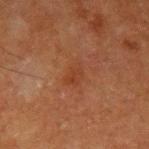Clinical impression: No biopsy was performed on this lesion — it was imaged during a full skin examination and was not determined to be concerning. Acquisition and patient details: A 15 mm close-up extracted from a 3D total-body photography capture. Imaged with cross-polarized lighting. A male patient aged around 75. Longest diameter approximately 3 mm. On the left upper arm. Automated tile analysis of the lesion measured a footprint of about 4 mm², a shape eccentricity near 0.8, and a shape-asymmetry score of about 0.25 (0 = symmetric). And it measured a lesion color around L≈32 a*≈22 b*≈29 in CIELAB, about 4 CIELAB-L* units darker than the surrounding skin, and a normalized lesion–skin contrast near 5. And it measured a border-irregularity rating of about 2.5/10 and a within-lesion color-variation index near 2/10. And it measured a nevus-likeness score of about 0/100 and a lesion-detection confidence of about 100/100.